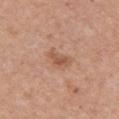Clinical impression: This lesion was catalogued during total-body skin photography and was not selected for biopsy. Clinical summary: About 3 mm across. An algorithmic analysis of the crop reported a lesion area of about 4 mm², a shape eccentricity near 0.8, and two-axis asymmetry of about 0.4. The software also gave roughly 9 lightness units darker than nearby skin. And it measured a border-irregularity index near 4.5/10, a within-lesion color-variation index near 2/10, and peripheral color asymmetry of about 0.5. A female subject, approximately 60 years of age. Cropped from a total-body skin-imaging series; the visible field is about 15 mm. Imaged with white-light lighting. On the chest.